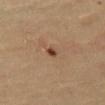notes: total-body-photography surveillance lesion; no biopsy
patient: in their 60s
location: the left thigh
image: ~15 mm crop, total-body skin-cancer survey
tile lighting: cross-polarized
TBP lesion metrics: an outline eccentricity of about 0.45 (0 = round, 1 = elongated) and a shape-asymmetry score of about 0.25 (0 = symmetric); a mean CIELAB color near L≈42 a*≈21 b*≈31, a lesion–skin lightness drop of about 13, and a normalized lesion–skin contrast near 10